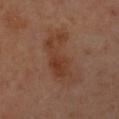Q: Was this lesion biopsied?
A: imaged on a skin check; not biopsied
Q: Lesion location?
A: the right forearm
Q: How large is the lesion?
A: ~7 mm (longest diameter)
Q: Patient demographics?
A: female, about 50 years old
Q: What is the imaging modality?
A: ~15 mm crop, total-body skin-cancer survey